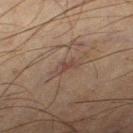The lesion was photographed on a routine skin check and not biopsied; there is no pathology result. A male subject in their mid-60s. The total-body-photography lesion software estimated a lesion area of about 3 mm², an eccentricity of roughly 0.9, and a shape-asymmetry score of about 0.45 (0 = symmetric). And it measured a mean CIELAB color near L≈34 a*≈14 b*≈19, roughly 5 lightness units darker than nearby skin, and a lesion-to-skin contrast of about 5.5 (normalized; higher = more distinct). The software also gave internal color variation of about 0 on a 0–10 scale and a peripheral color-asymmetry measure near 0. From the right thigh. A close-up tile cropped from a whole-body skin photograph, about 15 mm across. Approximately 3 mm at its widest. This is a cross-polarized tile.